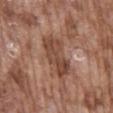workup=catalogued during a skin exam; not biopsied
patient=male, roughly 75 years of age
lighting=white-light illumination
site=the mid back
imaging modality=~15 mm crop, total-body skin-cancer survey